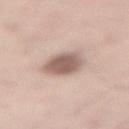• follow-up — catalogued during a skin exam; not biopsied
• size — ~4 mm (longest diameter)
• imaging modality — total-body-photography crop, ~15 mm field of view
• subject — male, aged 53–57
• site — the back